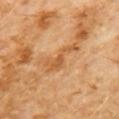<record>
<biopsy_status>not biopsied; imaged during a skin examination</biopsy_status>
<image>
  <source>total-body photography crop</source>
  <field_of_view_mm>15</field_of_view_mm>
</image>
<patient>
  <sex>male</sex>
  <age_approx>60</age_approx>
</patient>
<site>chest</site>
<lighting>cross-polarized</lighting>
<lesion_size>
  <long_diameter_mm_approx>5.0</long_diameter_mm_approx>
</lesion_size>
</record>